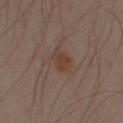Assessment: Recorded during total-body skin imaging; not selected for excision or biopsy. Context: A close-up tile cropped from a whole-body skin photograph, about 15 mm across. The lesion is located on the right upper arm. The patient is a male aged around 45. Imaged with cross-polarized lighting. The total-body-photography lesion software estimated a lesion area of about 4.5 mm² and a shape eccentricity near 0.65. It also reported a lesion–skin lightness drop of about 6 and a normalized border contrast of about 6.5. And it measured a border-irregularity index near 2/10 and peripheral color asymmetry of about 1. The software also gave an automated nevus-likeness rating near 60 out of 100 and a detector confidence of about 100 out of 100 that the crop contains a lesion.